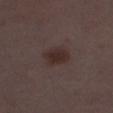The lesion was photographed on a routine skin check and not biopsied; there is no pathology result. Captured under white-light illumination. A female patient, about 30 years old. Automated tile analysis of the lesion measured an area of roughly 7 mm², an eccentricity of roughly 0.7, and two-axis asymmetry of about 0.2. It also reported a lesion color around L≈28 a*≈14 b*≈17 in CIELAB, roughly 7 lightness units darker than nearby skin, and a normalized lesion–skin contrast near 8. The software also gave a border-irregularity rating of about 1.5/10, internal color variation of about 2.5 on a 0–10 scale, and a peripheral color-asymmetry measure near 0.5. Cropped from a total-body skin-imaging series; the visible field is about 15 mm. Located on the leg.